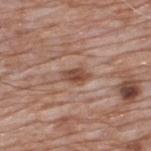Q: Is there a histopathology result?
A: imaged on a skin check; not biopsied
Q: How was the tile lit?
A: white-light
Q: What is the imaging modality?
A: total-body-photography crop, ~15 mm field of view
Q: What did automated image analysis measure?
A: a symmetry-axis asymmetry near 0.25; a lesion-detection confidence of about 100/100
Q: Lesion location?
A: the upper back
Q: Patient demographics?
A: male, aged around 60
Q: Lesion size?
A: about 3 mm